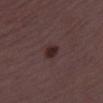Q: Was this lesion biopsied?
A: no biopsy performed (imaged during a skin exam)
Q: What is the anatomic site?
A: the right thigh
Q: What are the patient's age and sex?
A: female, aged 28 to 32
Q: How was this image acquired?
A: ~15 mm tile from a whole-body skin photo